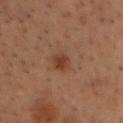Case summary:
– notes: imaged on a skin check; not biopsied
– subject: male, aged 53 to 57
– anatomic site: the chest
– acquisition: ~15 mm crop, total-body skin-cancer survey